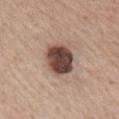{"biopsy_status": "not biopsied; imaged during a skin examination", "lighting": "white-light", "lesion_size": {"long_diameter_mm_approx": 4.5}, "site": "right upper arm", "image": {"source": "total-body photography crop", "field_of_view_mm": 15}, "patient": {"sex": "male", "age_approx": 75}}